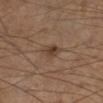Part of a total-body skin-imaging series; this lesion was reviewed on a skin check and was not flagged for biopsy. This image is a 15 mm lesion crop taken from a total-body photograph. The subject is a male roughly 55 years of age. Automated tile analysis of the lesion measured a lesion area of about 4 mm², an outline eccentricity of about 0.85 (0 = round, 1 = elongated), and a symmetry-axis asymmetry near 0.3. It also reported a border-irregularity index near 3/10, a color-variation rating of about 3/10, and radial color variation of about 1. The lesion is located on the left lower leg. Longest diameter approximately 3.5 mm. This is a cross-polarized tile.